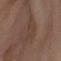A 15 mm close-up tile from a total-body photography series done for melanoma screening. Located on the front of the torso. Captured under cross-polarized illumination. Automated image analysis of the tile measured a nevus-likeness score of about 0/100 and a lesion-detection confidence of about 55/100. Measured at roughly 4.5 mm in maximum diameter. The patient is a female aged around 55.